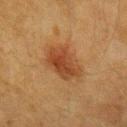Captured under cross-polarized illumination. Measured at roughly 5 mm in maximum diameter. A female patient, aged approximately 55. Automated tile analysis of the lesion measured two-axis asymmetry of about 0.2. The analysis additionally found about 9 CIELAB-L* units darker than the surrounding skin. It also reported a border-irregularity index near 2.5/10, a within-lesion color-variation index near 4/10, and peripheral color asymmetry of about 1.5. And it measured a nevus-likeness score of about 100/100 and a detector confidence of about 100 out of 100 that the crop contains a lesion. A lesion tile, about 15 mm wide, cut from a 3D total-body photograph. The lesion is on the right forearm.